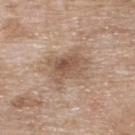Assessment:
The lesion was photographed on a routine skin check and not biopsied; there is no pathology result.
Clinical summary:
The lesion-visualizer software estimated a mean CIELAB color near L≈55 a*≈16 b*≈28 and a lesion–skin lightness drop of about 10. It also reported border irregularity of about 4 on a 0–10 scale and a within-lesion color-variation index near 5.5/10. And it measured a classifier nevus-likeness of about 0/100 and a lesion-detection confidence of about 100/100. Located on the upper back. The lesion's longest dimension is about 4.5 mm. The tile uses white-light illumination. A female patient, about 75 years old. Cropped from a total-body skin-imaging series; the visible field is about 15 mm.